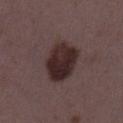Case summary:
• notes · no biopsy performed (imaged during a skin exam)
• tile lighting · white-light
• patient · female, in their mid-30s
• body site · the left thigh
• image source · ~15 mm tile from a whole-body skin photo
• TBP lesion metrics · an area of roughly 15 mm² and two-axis asymmetry of about 0.15; a lesion color around L≈26 a*≈16 b*≈15 in CIELAB and a normalized lesion–skin contrast near 12.5